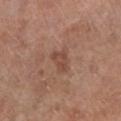Notes:
• follow-up: no biopsy performed (imaged during a skin exam)
• illumination: white-light
• acquisition: ~15 mm crop, total-body skin-cancer survey
• anatomic site: the left lower leg
• patient: male, aged 68 to 72
• TBP lesion metrics: a footprint of about 4 mm² and a symmetry-axis asymmetry near 0.35; an average lesion color of about L≈47 a*≈22 b*≈27 (CIELAB), a lesion–skin lightness drop of about 8, and a lesion-to-skin contrast of about 6 (normalized; higher = more distinct); a color-variation rating of about 2.5/10 and peripheral color asymmetry of about 1; an automated nevus-likeness rating near 15 out of 100 and lesion-presence confidence of about 100/100
• size: ≈3 mm